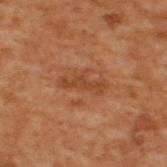Recorded during total-body skin imaging; not selected for excision or biopsy. Measured at roughly 4.5 mm in maximum diameter. The lesion is on the upper back. A 15 mm crop from a total-body photograph taken for skin-cancer surveillance. Imaged with cross-polarized lighting. A male subject, aged 68 to 72.